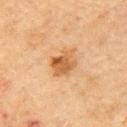biopsy status = imaged on a skin check; not biopsied | body site = the right upper arm | image = total-body-photography crop, ~15 mm field of view | subject = female, in their 80s.